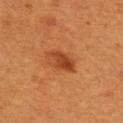workup: no biopsy performed (imaged during a skin exam)
image-analysis metrics: a shape eccentricity near 0.8 and a shape-asymmetry score of about 0.2 (0 = symmetric); a lesion color around L≈42 a*≈28 b*≈38 in CIELAB and a normalized border contrast of about 7.5
body site: the upper back
subject: male, aged 53 to 57
lesion diameter: about 4 mm
tile lighting: cross-polarized illumination
imaging modality: 15 mm crop, total-body photography